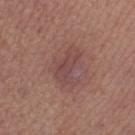| feature | finding |
|---|---|
| image-analysis metrics | a mean CIELAB color near L≈46 a*≈21 b*≈21 and roughly 7 lightness units darker than nearby skin |
| anatomic site | the right lower leg |
| illumination | white-light illumination |
| imaging modality | ~15 mm tile from a whole-body skin photo |
| patient | female, roughly 40 years of age |